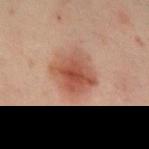The lesion was photographed on a routine skin check and not biopsied; there is no pathology result. A roughly 15 mm field-of-view crop from a total-body skin photograph. This is a cross-polarized tile. An algorithmic analysis of the crop reported a footprint of about 15 mm², a shape eccentricity near 0.45, and two-axis asymmetry of about 0.2. The analysis additionally found a normalized lesion–skin contrast near 8. It also reported border irregularity of about 2.5 on a 0–10 scale, internal color variation of about 5 on a 0–10 scale, and peripheral color asymmetry of about 1.5. Measured at roughly 4.5 mm in maximum diameter. The lesion is on the left forearm. A male subject, aged 48–52.